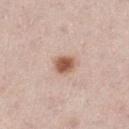Findings:
- lighting: white-light
- patient: male, in their mid-60s
- imaging modality: ~15 mm crop, total-body skin-cancer survey
- anatomic site: the right thigh